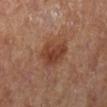  biopsy_status: not biopsied; imaged during a skin examination
  lesion_size:
    long_diameter_mm_approx: 4.5
  image:
    source: total-body photography crop
    field_of_view_mm: 15
  patient:
    sex: female
  site: left lower leg
  lighting: cross-polarized
  automated_metrics:
    color_variation_0_10: 3.5
    peripheral_color_asymmetry: 1.5